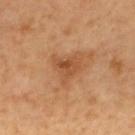Notes:
• follow-up — total-body-photography surveillance lesion; no biopsy
• patient — male, aged approximately 60
• illumination — cross-polarized
• body site — the upper back
• acquisition — 15 mm crop, total-body photography